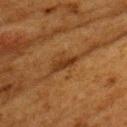| feature | finding |
|---|---|
| follow-up | no biopsy performed (imaged during a skin exam) |
| subject | female, aged around 50 |
| automated metrics | a border-irregularity rating of about 4/10, a within-lesion color-variation index near 2/10, and peripheral color asymmetry of about 0.5; a lesion-detection confidence of about 70/100 |
| site | the arm |
| tile lighting | cross-polarized illumination |
| imaging modality | ~15 mm tile from a whole-body skin photo |